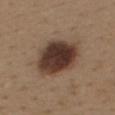This lesion was catalogued during total-body skin photography and was not selected for biopsy. A lesion tile, about 15 mm wide, cut from a 3D total-body photograph. A female subject aged approximately 40. Captured under white-light illumination. The lesion's longest dimension is about 6.5 mm. Automated tile analysis of the lesion measured a color-variation rating of about 6/10 and radial color variation of about 1.5. It also reported a lesion-detection confidence of about 100/100. On the back.